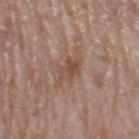Assessment:
The lesion was photographed on a routine skin check and not biopsied; there is no pathology result.
Image and clinical context:
This image is a 15 mm lesion crop taken from a total-body photograph. A female patient about 60 years old. The lesion is on the left thigh. About 4 mm across. Imaged with white-light lighting. Automated image analysis of the tile measured an area of roughly 5.5 mm² and a shape eccentricity near 0.8.